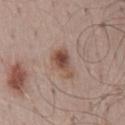Case summary:
- biopsy status: total-body-photography surveillance lesion; no biopsy
- image: ~15 mm tile from a whole-body skin photo
- subject: male, aged 48 to 52
- location: the mid back
- lesion diameter: about 4 mm